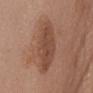Notes:
– workup · total-body-photography surveillance lesion; no biopsy
– anatomic site · the abdomen
– subject · female, aged 58–62
– acquisition · total-body-photography crop, ~15 mm field of view
– tile lighting · white-light illumination
– lesion diameter · ≈6.5 mm
– TBP lesion metrics · a mean CIELAB color near L≈47 a*≈20 b*≈28 and roughly 9 lightness units darker than nearby skin; a classifier nevus-likeness of about 5/100 and a lesion-detection confidence of about 100/100